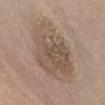Impression: The lesion was tiled from a total-body skin photograph and was not biopsied. Acquisition and patient details: A 15 mm close-up tile from a total-body photography series done for melanoma screening. On the abdomen. A male patient, roughly 75 years of age. An algorithmic analysis of the crop reported an average lesion color of about L≈53 a*≈13 b*≈25 (CIELAB) and about 9 CIELAB-L* units darker than the surrounding skin. Approximately 10.5 mm at its widest.